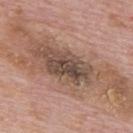Q: Was a biopsy performed?
A: no biopsy performed (imaged during a skin exam)
Q: Who is the patient?
A: male, aged around 75
Q: How was this image acquired?
A: ~15 mm tile from a whole-body skin photo
Q: How large is the lesion?
A: ~17 mm (longest diameter)
Q: How was the tile lit?
A: white-light illumination
Q: What did automated image analysis measure?
A: a mean CIELAB color near L≈54 a*≈18 b*≈28 and roughly 10 lightness units darker than nearby skin; border irregularity of about 8 on a 0–10 scale and a color-variation rating of about 8/10; a detector confidence of about 90 out of 100 that the crop contains a lesion
Q: Where on the body is the lesion?
A: the upper back